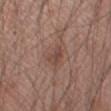follow-up: imaged on a skin check; not biopsied | location: the left forearm | tile lighting: white-light | image source: 15 mm crop, total-body photography | subject: male, roughly 45 years of age | diameter: ≈2.5 mm | automated lesion analysis: an area of roughly 4 mm² and a shape-asymmetry score of about 0.35 (0 = symmetric); an average lesion color of about L≈45 a*≈19 b*≈24 (CIELAB) and a normalized lesion–skin contrast near 6; a nevus-likeness score of about 15/100.